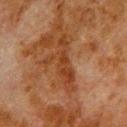The lesion was photographed on a routine skin check and not biopsied; there is no pathology result. The lesion is located on the back. An algorithmic analysis of the crop reported roughly 7 lightness units darker than nearby skin and a lesion-to-skin contrast of about 7 (normalized; higher = more distinct). The software also gave a border-irregularity rating of about 10/10, a color-variation rating of about 2.5/10, and peripheral color asymmetry of about 0.5. A male patient, aged 78–82. A lesion tile, about 15 mm wide, cut from a 3D total-body photograph. The tile uses cross-polarized illumination.